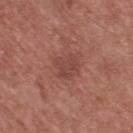Case summary:
- workup: imaged on a skin check; not biopsied
- diameter: about 3 mm
- anatomic site: the upper back
- subject: male, about 55 years old
- image: 15 mm crop, total-body photography
- lighting: white-light